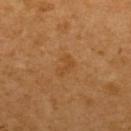Q: Was this lesion biopsied?
A: catalogued during a skin exam; not biopsied
Q: What are the patient's age and sex?
A: female, aged approximately 55
Q: How was the tile lit?
A: cross-polarized illumination
Q: What kind of image is this?
A: 15 mm crop, total-body photography
Q: Automated lesion metrics?
A: a footprint of about 3.5 mm², an eccentricity of roughly 0.8, and a symmetry-axis asymmetry near 0.45; a border-irregularity rating of about 4.5/10 and internal color variation of about 1 on a 0–10 scale
Q: Lesion location?
A: the upper back